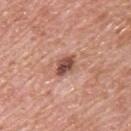Imaged with white-light lighting. On the back. Longest diameter approximately 3 mm. Cropped from a total-body skin-imaging series; the visible field is about 15 mm. Automated image analysis of the tile measured a border-irregularity rating of about 2.5/10, a color-variation rating of about 4/10, and a peripheral color-asymmetry measure near 1. A male patient, aged around 60.